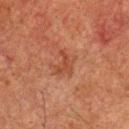| key | value |
|---|---|
| subject | male, in their 60s |
| image source | ~15 mm tile from a whole-body skin photo |
| site | the head or neck |